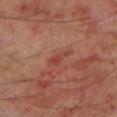Longest diameter approximately 3 mm.
Located on the left lower leg.
A male subject, in their 70s.
This image is a 15 mm lesion crop taken from a total-body photograph.
An algorithmic analysis of the crop reported an average lesion color of about L≈42 a*≈29 b*≈28 (CIELAB), roughly 7 lightness units darker than nearby skin, and a lesion-to-skin contrast of about 5.5 (normalized; higher = more distinct). And it measured a border-irregularity index near 5/10, internal color variation of about 0.5 on a 0–10 scale, and peripheral color asymmetry of about 0. It also reported a classifier nevus-likeness of about 0/100 and lesion-presence confidence of about 100/100.
Imaged with cross-polarized lighting.